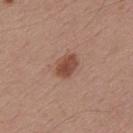The lesion was tiled from a total-body skin photograph and was not biopsied. Cropped from a whole-body photographic skin survey; the tile spans about 15 mm. The patient is a male aged 53 to 57. Imaged with white-light lighting. From the mid back.